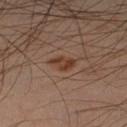Image and clinical context:
Captured under cross-polarized illumination. A male patient in their 50s. Measured at roughly 3 mm in maximum diameter. A 15 mm crop from a total-body photograph taken for skin-cancer surveillance. Located on the left lower leg.